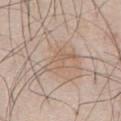Q: Was a biopsy performed?
A: total-body-photography surveillance lesion; no biopsy
Q: Patient demographics?
A: male, approximately 60 years of age
Q: Lesion size?
A: ~4 mm (longest diameter)
Q: Illumination type?
A: white-light illumination
Q: What is the imaging modality?
A: total-body-photography crop, ~15 mm field of view
Q: What is the anatomic site?
A: the abdomen
Q: What did automated image analysis measure?
A: a lesion color around L≈60 a*≈16 b*≈28 in CIELAB, about 6 CIELAB-L* units darker than the surrounding skin, and a normalized lesion–skin contrast near 5; a border-irregularity index near 6.5/10 and a peripheral color-asymmetry measure near 0.5; an automated nevus-likeness rating near 0 out of 100 and a lesion-detection confidence of about 90/100